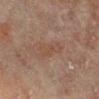Part of a total-body skin-imaging series; this lesion was reviewed on a skin check and was not flagged for biopsy. This is a cross-polarized tile. A lesion tile, about 15 mm wide, cut from a 3D total-body photograph. A female subject, in their 80s. Approximately 4 mm at its widest. The lesion is on the right lower leg.